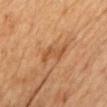No biopsy was performed on this lesion — it was imaged during a full skin examination and was not determined to be concerning. A male patient, in their 70s. Cropped from a total-body skin-imaging series; the visible field is about 15 mm. Approximately 4 mm at its widest. Located on the chest. The tile uses cross-polarized illumination.